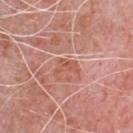workup: imaged on a skin check; not biopsied
automated metrics: border irregularity of about 6.5 on a 0–10 scale, a within-lesion color-variation index near 1.5/10, and radial color variation of about 0.5; a nevus-likeness score of about 0/100
lesion diameter: about 3 mm
subject: male, approximately 80 years of age
body site: the chest
imaging modality: ~15 mm crop, total-body skin-cancer survey
lighting: white-light